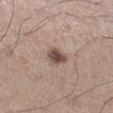No biopsy was performed on this lesion — it was imaged during a full skin examination and was not determined to be concerning. The total-body-photography lesion software estimated border irregularity of about 2 on a 0–10 scale and a within-lesion color-variation index near 4.5/10. Approximately 2.5 mm at its widest. The lesion is located on the right lower leg. Imaged with white-light lighting. A male patient about 60 years old. Cropped from a total-body skin-imaging series; the visible field is about 15 mm.